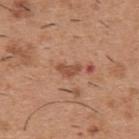lesion diameter=≈3 mm | anatomic site=the back | TBP lesion metrics=a lesion color around L≈50 a*≈23 b*≈32 in CIELAB, a lesion–skin lightness drop of about 10, and a normalized border contrast of about 7; a border-irregularity index near 4.5/10, internal color variation of about 1 on a 0–10 scale, and a peripheral color-asymmetry measure near 0.5 | acquisition=15 mm crop, total-body photography | subject=male, aged 38 to 42 | illumination=white-light illumination.